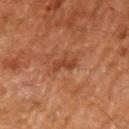  lesion_size:
    long_diameter_mm_approx: 2.5
  automated_metrics:
    area_mm2_approx: 3.5
    eccentricity: 0.8
    vs_skin_darker_L: 7.0
  patient:
    sex: male
    age_approx: 60
  lighting: cross-polarized
  site: left upper arm
  image:
    source: total-body photography crop
    field_of_view_mm: 15